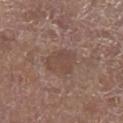Case summary:
- biopsy status — no biopsy performed (imaged during a skin exam)
- automated lesion analysis — an eccentricity of roughly 0.65 and a shape-asymmetry score of about 0.25 (0 = symmetric)
- lighting — white-light
- location — the left lower leg
- patient — male, approximately 75 years of age
- acquisition — 15 mm crop, total-body photography
- diameter — about 4 mm